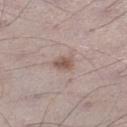<tbp_lesion>
  <biopsy_status>not biopsied; imaged during a skin examination</biopsy_status>
  <lesion_size>
    <long_diameter_mm_approx>2.5</long_diameter_mm_approx>
  </lesion_size>
  <image>
    <source>total-body photography crop</source>
    <field_of_view_mm>15</field_of_view_mm>
  </image>
  <site>left lower leg</site>
  <patient>
    <sex>male</sex>
    <age_approx>70</age_approx>
  </patient>
  <automated_metrics>
    <area_mm2_approx>4.0</area_mm2_approx>
    <shape_asymmetry>0.25</shape_asymmetry>
    <nevus_likeness_0_100>70</nevus_likeness_0_100>
  </automated_metrics>
  <lighting>white-light</lighting>
</tbp_lesion>